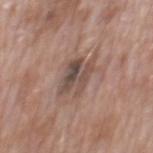  biopsy_status: not biopsied; imaged during a skin examination
  image:
    source: total-body photography crop
    field_of_view_mm: 15
  automated_metrics:
    area_mm2_approx: 11.0
    eccentricity: 0.7
    shape_asymmetry: 0.2
    color_variation_0_10: 7.5
    peripheral_color_asymmetry: 2.5
    lesion_detection_confidence_0_100: 95
  lesion_size:
    long_diameter_mm_approx: 4.5
  site: mid back
  patient:
    sex: male
    age_approx: 70